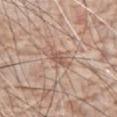Clinical impression:
Imaged during a routine full-body skin examination; the lesion was not biopsied and no histopathology is available.
Acquisition and patient details:
The tile uses white-light illumination. A region of skin cropped from a whole-body photographic capture, roughly 15 mm wide. The lesion is on the abdomen. The lesion's longest dimension is about 3.5 mm. A male subject, aged around 80.